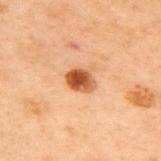Acquisition and patient details: A male patient aged around 70. The lesion is located on the upper back. Cropped from a total-body skin-imaging series; the visible field is about 15 mm.